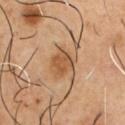Part of a total-body skin-imaging series; this lesion was reviewed on a skin check and was not flagged for biopsy.